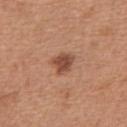Findings:
• workup · total-body-photography surveillance lesion; no biopsy
• location · the abdomen
• acquisition · 15 mm crop, total-body photography
• patient · male, in their mid- to late 60s